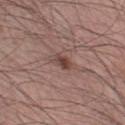<tbp_lesion>
  <biopsy_status>not biopsied; imaged during a skin examination</biopsy_status>
  <lighting>white-light</lighting>
  <site>left lower leg</site>
  <image>
    <source>total-body photography crop</source>
    <field_of_view_mm>15</field_of_view_mm>
  </image>
  <patient>
    <sex>male</sex>
    <age_approx>35</age_approx>
  </patient>
</tbp_lesion>